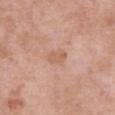Notes:
- notes · no biopsy performed (imaged during a skin exam)
- lesion size · ≈2.5 mm
- imaging modality · 15 mm crop, total-body photography
- illumination · white-light illumination
- body site · the left upper arm
- patient · male, in their mid-50s